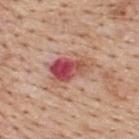{
  "biopsy_status": "not biopsied; imaged during a skin examination",
  "image": {
    "source": "total-body photography crop",
    "field_of_view_mm": 15
  },
  "lighting": "white-light",
  "patient": {
    "sex": "male",
    "age_approx": 60
  },
  "site": "back",
  "automated_metrics": {
    "area_mm2_approx": 12.0,
    "eccentricity": 0.85,
    "shape_asymmetry": 0.35,
    "cielab_L": 52,
    "cielab_a": 29,
    "cielab_b": 26,
    "vs_skin_darker_L": 14.0,
    "vs_skin_contrast_norm": 9.5,
    "border_irregularity_0_10": 4.0,
    "color_variation_0_10": 10.0,
    "peripheral_color_asymmetry": 4.5,
    "nevus_likeness_0_100": 0,
    "lesion_detection_confidence_0_100": 100
  },
  "lesion_size": {
    "long_diameter_mm_approx": 5.0
  }
}